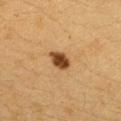Part of a total-body skin-imaging series; this lesion was reviewed on a skin check and was not flagged for biopsy. A 15 mm close-up tile from a total-body photography series done for melanoma screening. The lesion-visualizer software estimated a mean CIELAB color near L≈42 a*≈21 b*≈36 and about 17 CIELAB-L* units darker than the surrounding skin. It also reported a classifier nevus-likeness of about 100/100 and a detector confidence of about 100 out of 100 that the crop contains a lesion. A female subject, aged around 30. The lesion is on the right forearm.